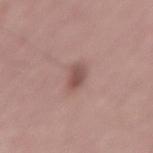notes: no biopsy performed (imaged during a skin exam) | imaging modality: ~15 mm tile from a whole-body skin photo | patient: male, in their mid-50s.